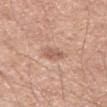Case summary:
– lighting · white-light
– site · the left thigh
– patient · male, aged approximately 65
– image-analysis metrics · a lesion area of about 4 mm² and two-axis asymmetry of about 0.3; a lesion color around L≈58 a*≈21 b*≈28 in CIELAB, roughly 9 lightness units darker than nearby skin, and a normalized lesion–skin contrast near 6; a border-irregularity index near 2.5/10, a within-lesion color-variation index near 2/10, and a peripheral color-asymmetry measure near 0.5; a nevus-likeness score of about 5/100 and a detector confidence of about 100 out of 100 that the crop contains a lesion
– size · about 2.5 mm
– image · total-body-photography crop, ~15 mm field of view